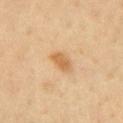biopsy status = imaged on a skin check; not biopsied.